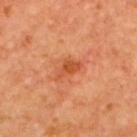notes: no biopsy performed (imaged during a skin exam) | automated lesion analysis: a lesion color around L≈54 a*≈33 b*≈42 in CIELAB, about 9 CIELAB-L* units darker than the surrounding skin, and a lesion-to-skin contrast of about 7 (normalized; higher = more distinct); border irregularity of about 3.5 on a 0–10 scale, internal color variation of about 3.5 on a 0–10 scale, and radial color variation of about 1 | tile lighting: cross-polarized illumination | diameter: ≈3.5 mm | subject: male, aged 63–67 | anatomic site: the upper back | image source: total-body-photography crop, ~15 mm field of view.